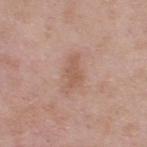biopsy_status: not biopsied; imaged during a skin examination
image:
  source: total-body photography crop
  field_of_view_mm: 15
site: upper back
patient:
  sex: male
  age_approx: 55